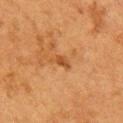{"biopsy_status": "not biopsied; imaged during a skin examination", "patient": {"sex": "female", "age_approx": 50}, "site": "chest", "image": {"source": "total-body photography crop", "field_of_view_mm": 15}, "lighting": "cross-polarized"}